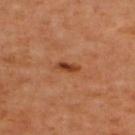No biopsy was performed on this lesion — it was imaged during a full skin examination and was not determined to be concerning. A male subject, aged 63 to 67. This is a cross-polarized tile. About 2.5 mm across. Cropped from a total-body skin-imaging series; the visible field is about 15 mm. On the upper back.